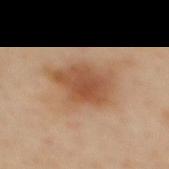<record>
  <lighting>cross-polarized</lighting>
  <site>upper back</site>
  <patient>
    <sex>female</sex>
    <age_approx>40</age_approx>
  </patient>
  <lesion_size>
    <long_diameter_mm_approx>6.5</long_diameter_mm_approx>
  </lesion_size>
  <image>
    <source>total-body photography crop</source>
    <field_of_view_mm>15</field_of_view_mm>
  </image>
</record>